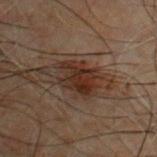Q: Was this lesion biopsied?
A: catalogued during a skin exam; not biopsied
Q: Illumination type?
A: cross-polarized
Q: Lesion location?
A: the chest
Q: What is the imaging modality?
A: 15 mm crop, total-body photography
Q: Who is the patient?
A: male, aged 48–52
Q: Lesion size?
A: about 4 mm